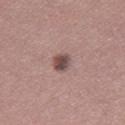Captured during whole-body skin photography for melanoma surveillance; the lesion was not biopsied.
Automated tile analysis of the lesion measured a lesion area of about 4.5 mm², a shape eccentricity near 0.6, and two-axis asymmetry of about 0.15. It also reported a border-irregularity index near 1.5/10 and a peripheral color-asymmetry measure near 1.5.
About 2.5 mm across.
This is a white-light tile.
A female patient, aged 33–37.
The lesion is located on the left leg.
A lesion tile, about 15 mm wide, cut from a 3D total-body photograph.